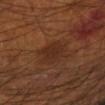Assessment:
No biopsy was performed on this lesion — it was imaged during a full skin examination and was not determined to be concerning.
Image and clinical context:
The total-body-photography lesion software estimated an area of roughly 9 mm², a shape eccentricity near 0.7, and two-axis asymmetry of about 0.2. The patient is a male about 55 years old. About 4 mm across. A 15 mm close-up extracted from a 3D total-body photography capture. The tile uses cross-polarized illumination. Located on the right forearm.